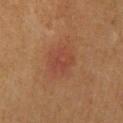  biopsy_status: not biopsied; imaged during a skin examination
  image:
    source: total-body photography crop
    field_of_view_mm: 15
  site: upper back
  patient:
    sex: male
    age_approx: 60